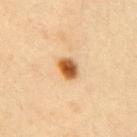Imaged during a routine full-body skin examination; the lesion was not biopsied and no histopathology is available.
From the arm.
Captured under cross-polarized illumination.
An algorithmic analysis of the crop reported border irregularity of about 1.5 on a 0–10 scale, a color-variation rating of about 6/10, and a peripheral color-asymmetry measure near 1.5. The software also gave an automated nevus-likeness rating near 100 out of 100.
Measured at roughly 3 mm in maximum diameter.
A lesion tile, about 15 mm wide, cut from a 3D total-body photograph.
The patient is a female aged around 30.